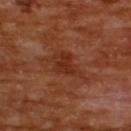{"biopsy_status": "not biopsied; imaged during a skin examination", "patient": {"sex": "male", "age_approx": 65}, "site": "upper back", "lighting": "cross-polarized", "image": {"source": "total-body photography crop", "field_of_view_mm": 15}, "lesion_size": {"long_diameter_mm_approx": 5.0}}